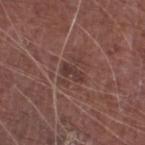site: left lower leg
patient:
  sex: male
  age_approx: 75
automated_metrics:
  eccentricity: 0.9
  shape_asymmetry: 0.3
  border_irregularity_0_10: 4.0
  peripheral_color_asymmetry: 0.0
lesion_size:
  long_diameter_mm_approx: 3.0
lighting: white-light
image:
  source: total-body photography crop
  field_of_view_mm: 15